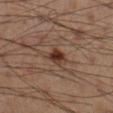Q: Was a biopsy performed?
A: no biopsy performed (imaged during a skin exam)
Q: How was the tile lit?
A: cross-polarized illumination
Q: What kind of image is this?
A: total-body-photography crop, ~15 mm field of view
Q: What is the lesion's diameter?
A: about 2.5 mm
Q: Automated lesion metrics?
A: an eccentricity of roughly 0.55
Q: What are the patient's age and sex?
A: male, approximately 50 years of age
Q: Where on the body is the lesion?
A: the right lower leg